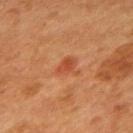Case summary:
– site — the mid back
– lighting — cross-polarized
– size — ≈2.5 mm
– subject — female, aged 38–42
– image — 15 mm crop, total-body photography